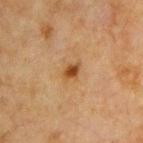Assessment: Recorded during total-body skin imaging; not selected for excision or biopsy. Image and clinical context: This image is a 15 mm lesion crop taken from a total-body photograph. The lesion is located on the chest. A male subject aged around 65.